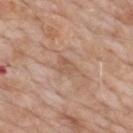  biopsy_status: not biopsied; imaged during a skin examination
  patient:
    sex: male
    age_approx: 60
  lighting: white-light
  automated_metrics:
    cielab_L: 56
    cielab_a: 19
    cielab_b: 30
    vs_skin_darker_L: 6.0
    vs_skin_contrast_norm: 4.5
    border_irregularity_0_10: 3.0
    color_variation_0_10: 2.0
    peripheral_color_asymmetry: 1.0
  image:
    source: total-body photography crop
    field_of_view_mm: 15
  site: back
  lesion_size:
    long_diameter_mm_approx: 2.5